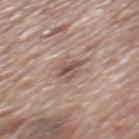The lesion was tiled from a total-body skin photograph and was not biopsied.
This is a white-light tile.
A male subject, aged 68–72.
The lesion-visualizer software estimated a nevus-likeness score of about 25/100 and a lesion-detection confidence of about 100/100.
Located on the back.
The recorded lesion diameter is about 2.5 mm.
Cropped from a whole-body photographic skin survey; the tile spans about 15 mm.